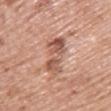Part of a total-body skin-imaging series; this lesion was reviewed on a skin check and was not flagged for biopsy.
A 15 mm close-up extracted from a 3D total-body photography capture.
A female subject roughly 60 years of age.
The lesion is located on the chest.
Captured under white-light illumination.